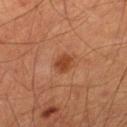Recorded during total-body skin imaging; not selected for excision or biopsy. The lesion-visualizer software estimated a footprint of about 4 mm², a shape eccentricity near 0.4, and two-axis asymmetry of about 0.2. And it measured a lesion color around L≈38 a*≈25 b*≈32 in CIELAB and about 10 CIELAB-L* units darker than the surrounding skin. It also reported a color-variation rating of about 1.5/10 and peripheral color asymmetry of about 0.5. About 2 mm across. From the left thigh. A male subject roughly 65 years of age. A lesion tile, about 15 mm wide, cut from a 3D total-body photograph. This is a cross-polarized tile.